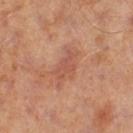{
  "biopsy_status": "not biopsied; imaged during a skin examination",
  "automated_metrics": {
    "area_mm2_approx": 8.5,
    "eccentricity": 0.85,
    "shape_asymmetry": 0.4,
    "cielab_L": 49,
    "cielab_a": 23,
    "cielab_b": 28,
    "vs_skin_darker_L": 7.0,
    "nevus_likeness_0_100": 0,
    "lesion_detection_confidence_0_100": 100
  },
  "patient": {
    "sex": "male",
    "age_approx": 60
  },
  "site": "left thigh",
  "image": {
    "source": "total-body photography crop",
    "field_of_view_mm": 15
  },
  "lesion_size": {
    "long_diameter_mm_approx": 5.0
  }
}